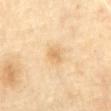Q: Was this lesion biopsied?
A: no biopsy performed (imaged during a skin exam)
Q: Illumination type?
A: cross-polarized illumination
Q: What is the lesion's diameter?
A: about 2 mm
Q: How was this image acquired?
A: 15 mm crop, total-body photography
Q: Patient demographics?
A: male, aged 68 to 72
Q: What is the anatomic site?
A: the abdomen